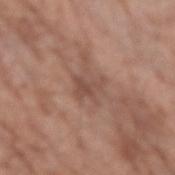{
  "biopsy_status": "not biopsied; imaged during a skin examination",
  "patient": {
    "sex": "male",
    "age_approx": 70
  },
  "site": "left forearm",
  "image": {
    "source": "total-body photography crop",
    "field_of_view_mm": 15
  },
  "lighting": "white-light",
  "automated_metrics": {
    "area_mm2_approx": 4.0,
    "eccentricity": 0.7,
    "shape_asymmetry": 0.45,
    "nevus_likeness_0_100": 0,
    "lesion_detection_confidence_0_100": 95
  },
  "lesion_size": {
    "long_diameter_mm_approx": 3.0
  }
}